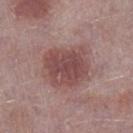| feature | finding |
|---|---|
| workup | no biopsy performed (imaged during a skin exam) |
| imaging modality | 15 mm crop, total-body photography |
| automated metrics | a footprint of about 20 mm², a shape eccentricity near 0.5, and two-axis asymmetry of about 0.2 |
| lesion size | ≈5 mm |
| patient | male, roughly 75 years of age |
| location | the left lower leg |